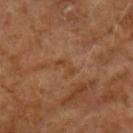Image and clinical context:
A male subject, in their mid- to late 50s. On the left upper arm. This is a cross-polarized tile. A 15 mm close-up tile from a total-body photography series done for melanoma screening.